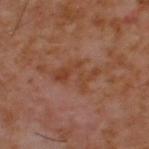Impression: Recorded during total-body skin imaging; not selected for excision or biopsy. Context: A region of skin cropped from a whole-body photographic capture, roughly 15 mm wide. Imaged with cross-polarized lighting. From the upper back. The patient is a male aged around 60. The lesion's longest dimension is about 4.5 mm. The total-body-photography lesion software estimated a border-irregularity rating of about 8.5/10, a color-variation rating of about 1/10, and radial color variation of about 0.5. It also reported a nevus-likeness score of about 0/100 and lesion-presence confidence of about 100/100.